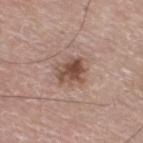Image and clinical context:
A 15 mm close-up tile from a total-body photography series done for melanoma screening. The tile uses white-light illumination. From the right thigh. A male patient aged around 75. The lesion-visualizer software estimated a footprint of about 7 mm² and a shape eccentricity near 0.55. The software also gave an average lesion color of about L≈49 a*≈19 b*≈25 (CIELAB), a lesion–skin lightness drop of about 12, and a normalized lesion–skin contrast near 9. And it measured a border-irregularity index near 3/10, a within-lesion color-variation index near 6/10, and a peripheral color-asymmetry measure near 2.5. It also reported a classifier nevus-likeness of about 70/100 and lesion-presence confidence of about 100/100.